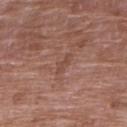This lesion was catalogued during total-body skin photography and was not selected for biopsy.
A region of skin cropped from a whole-body photographic capture, roughly 15 mm wide.
The patient is a female aged around 70.
The lesion is located on the right upper arm.
Automated image analysis of the tile measured about 6 CIELAB-L* units darker than the surrounding skin and a normalized border contrast of about 5. The software also gave a border-irregularity index near 4/10, a within-lesion color-variation index near 0/10, and radial color variation of about 0. The software also gave lesion-presence confidence of about 70/100.
Longest diameter approximately 3 mm.
Imaged with white-light lighting.